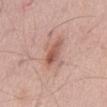Recorded during total-body skin imaging; not selected for excision or biopsy.
Approximately 4 mm at its widest.
Captured under white-light illumination.
This image is a 15 mm lesion crop taken from a total-body photograph.
On the abdomen.
A female patient aged approximately 65.